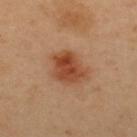Part of a total-body skin-imaging series; this lesion was reviewed on a skin check and was not flagged for biopsy.